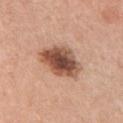Clinical impression: Recorded during total-body skin imaging; not selected for excision or biopsy. Background: Imaged with white-light lighting. From the left upper arm. A 15 mm close-up extracted from a 3D total-body photography capture. The patient is a female aged 58–62. The lesion's longest dimension is about 5.5 mm.